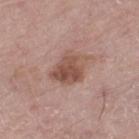{"lighting": "white-light", "automated_metrics": {"color_variation_0_10": 5.0, "peripheral_color_asymmetry": 2.0, "nevus_likeness_0_100": 20, "lesion_detection_confidence_0_100": 100}, "lesion_size": {"long_diameter_mm_approx": 4.0}, "site": "left thigh", "image": {"source": "total-body photography crop", "field_of_view_mm": 15}, "patient": {"sex": "male", "age_approx": 70}}